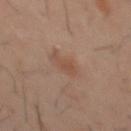Acquisition and patient details:
A 15 mm crop from a total-body photograph taken for skin-cancer surveillance. A male subject aged 38 to 42. Automated image analysis of the tile measured an area of roughly 3 mm² and a shape-asymmetry score of about 0.35 (0 = symmetric). It also reported a lesion color around L≈47 a*≈18 b*≈27 in CIELAB, a lesion–skin lightness drop of about 7, and a lesion-to-skin contrast of about 5.5 (normalized; higher = more distinct). The software also gave a nevus-likeness score of about 15/100 and lesion-presence confidence of about 100/100. From the mid back. Approximately 3 mm at its widest.